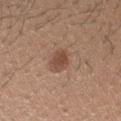This is a white-light tile.
About 3 mm across.
The lesion is on the head or neck.
A 15 mm close-up extracted from a 3D total-body photography capture.
A male subject, aged approximately 25.